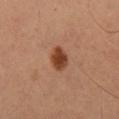Captured under cross-polarized illumination.
A region of skin cropped from a whole-body photographic capture, roughly 15 mm wide.
From the leg.
Longest diameter approximately 3 mm.
A male subject, approximately 50 years of age.